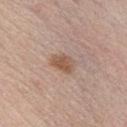<record>
  <biopsy_status>not biopsied; imaged during a skin examination</biopsy_status>
  <lighting>white-light</lighting>
  <lesion_size>
    <long_diameter_mm_approx>3.0</long_diameter_mm_approx>
  </lesion_size>
  <site>chest</site>
  <image>
    <source>total-body photography crop</source>
    <field_of_view_mm>15</field_of_view_mm>
  </image>
  <patient>
    <sex>male</sex>
    <age_approx>35</age_approx>
  </patient>
</record>